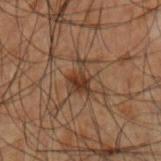notes = no biopsy performed (imaged during a skin exam) | TBP lesion metrics = a border-irregularity rating of about 5.5/10 and radial color variation of about 1; a nevus-likeness score of about 75/100 and lesion-presence confidence of about 95/100 | acquisition = ~15 mm tile from a whole-body skin photo | subject = male, aged 48 to 52 | size = ~3.5 mm (longest diameter) | location = the right forearm.